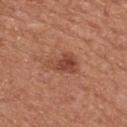Imaged during a routine full-body skin examination; the lesion was not biopsied and no histopathology is available. Automated tile analysis of the lesion measured a lesion–skin lightness drop of about 10 and a lesion-to-skin contrast of about 7.5 (normalized; higher = more distinct). The software also gave a border-irregularity rating of about 3.5/10, a within-lesion color-variation index near 5/10, and a peripheral color-asymmetry measure near 2. This image is a 15 mm lesion crop taken from a total-body photograph. A male subject in their mid- to late 60s. Longest diameter approximately 4 mm. On the mid back. Captured under white-light illumination.